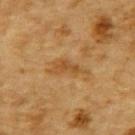biopsy status: total-body-photography surveillance lesion; no biopsy
image: ~15 mm tile from a whole-body skin photo
illumination: cross-polarized
location: the back
lesion size: about 4.5 mm
subject: male, aged approximately 85 or older
TBP lesion metrics: an area of roughly 5.5 mm², a shape eccentricity near 0.9, and two-axis asymmetry of about 0.45; a border-irregularity rating of about 5.5/10 and peripheral color asymmetry of about 0.5